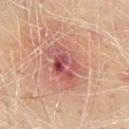{
  "lighting": "white-light",
  "site": "upper back",
  "image": {
    "source": "total-body photography crop",
    "field_of_view_mm": 15
  },
  "patient": {
    "sex": "male",
    "age_approx": 65
  }
}